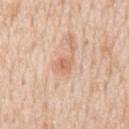Recorded during total-body skin imaging; not selected for excision or biopsy. Automated tile analysis of the lesion measured an area of roughly 4 mm² and two-axis asymmetry of about 0.25. The analysis additionally found an average lesion color of about L≈66 a*≈21 b*≈33 (CIELAB) and a normalized border contrast of about 6. The analysis additionally found border irregularity of about 2 on a 0–10 scale and radial color variation of about 1. The software also gave a classifier nevus-likeness of about 35/100. About 2.5 mm across. On the back. A 15 mm close-up extracted from a 3D total-body photography capture. The subject is a male approximately 60 years of age.